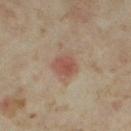A 15 mm close-up extracted from a 3D total-body photography capture. A female subject aged 33 to 37. Located on the right thigh. The lesion's longest dimension is about 3 mm.